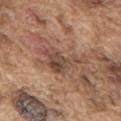  biopsy_status: not biopsied; imaged during a skin examination
  site: upper back
  lighting: white-light
  image:
    source: total-body photography crop
    field_of_view_mm: 15
  patient:
    sex: male
    age_approx: 75
  lesion_size:
    long_diameter_mm_approx: 5.5
  automated_metrics:
    cielab_L: 47
    cielab_a: 19
    cielab_b: 26
    vs_skin_darker_L: 9.0
    vs_skin_contrast_norm: 7.5